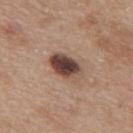Impression:
Part of a total-body skin-imaging series; this lesion was reviewed on a skin check and was not flagged for biopsy.
Acquisition and patient details:
A female subject aged 38 to 42. The tile uses white-light illumination. The lesion-visualizer software estimated peripheral color asymmetry of about 3. It also reported a nevus-likeness score of about 50/100 and a lesion-detection confidence of about 100/100. On the back. About 4.5 mm across. A 15 mm close-up tile from a total-body photography series done for melanoma screening.